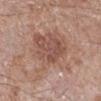notes: imaged on a skin check; not biopsied
tile lighting: white-light illumination
patient: male, in their 60s
lesion size: ~6.5 mm (longest diameter)
location: the right lower leg
acquisition: total-body-photography crop, ~15 mm field of view